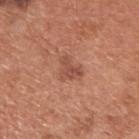Captured during whole-body skin photography for melanoma surveillance; the lesion was not biopsied. An algorithmic analysis of the crop reported a footprint of about 4.5 mm², an outline eccentricity of about 0.65 (0 = round, 1 = elongated), and a symmetry-axis asymmetry near 0.6. The software also gave a lesion color around L≈50 a*≈25 b*≈30 in CIELAB and about 9 CIELAB-L* units darker than the surrounding skin. The software also gave a border-irregularity index near 6.5/10 and a peripheral color-asymmetry measure near 0.5. It also reported a classifier nevus-likeness of about 15/100 and a lesion-detection confidence of about 100/100. A male subject in their mid-60s. The recorded lesion diameter is about 3 mm. Captured under white-light illumination. This image is a 15 mm lesion crop taken from a total-body photograph. On the upper back.